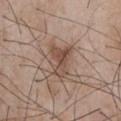Assessment:
Recorded during total-body skin imaging; not selected for excision or biopsy.
Background:
From the chest. A 15 mm crop from a total-body photograph taken for skin-cancer surveillance. A male patient about 50 years old. Automated tile analysis of the lesion measured border irregularity of about 6 on a 0–10 scale and internal color variation of about 4.5 on a 0–10 scale. Captured under white-light illumination. The recorded lesion diameter is about 4.5 mm.